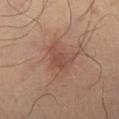The lesion was tiled from a total-body skin photograph and was not biopsied. The lesion's longest dimension is about 3.5 mm. On the right lower leg. This is a cross-polarized tile. The total-body-photography lesion software estimated a lesion color around L≈44 a*≈20 b*≈24 in CIELAB, about 7 CIELAB-L* units darker than the surrounding skin, and a normalized border contrast of about 5.5. It also reported a border-irregularity index near 3.5/10, internal color variation of about 2 on a 0–10 scale, and peripheral color asymmetry of about 0.5. A male patient aged 63–67. A lesion tile, about 15 mm wide, cut from a 3D total-body photograph.